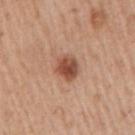Recorded during total-body skin imaging; not selected for excision or biopsy.
Approximately 3 mm at its widest.
Imaged with white-light lighting.
The lesion is located on the right upper arm.
A 15 mm close-up extracted from a 3D total-body photography capture.
A male subject in their mid-50s.